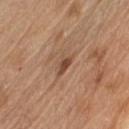Assessment: The lesion was tiled from a total-body skin photograph and was not biopsied. Background: The lesion is located on the right upper arm. About 2.5 mm across. A male patient aged approximately 70. A 15 mm crop from a total-body photograph taken for skin-cancer surveillance.